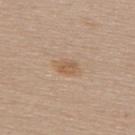Clinical impression: Part of a total-body skin-imaging series; this lesion was reviewed on a skin check and was not flagged for biopsy. Background: The lesion is located on the upper back. A 15 mm crop from a total-body photograph taken for skin-cancer surveillance. The patient is a female aged approximately 60.